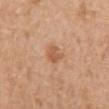Assessment: Imaged during a routine full-body skin examination; the lesion was not biopsied and no histopathology is available. Clinical summary: This is a white-light tile. A 15 mm close-up extracted from a 3D total-body photography capture. About 2.5 mm across. A male patient aged approximately 70. Located on the mid back.